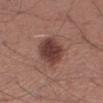<tbp_lesion>
<biopsy_status>not biopsied; imaged during a skin examination</biopsy_status>
</tbp_lesion>